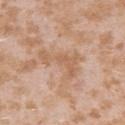The lesion was tiled from a total-body skin photograph and was not biopsied. Located on the arm. The tile uses white-light illumination. The lesion's longest dimension is about 5 mm. A lesion tile, about 15 mm wide, cut from a 3D total-body photograph. A female subject in their mid-20s. The lesion-visualizer software estimated a lesion area of about 9.5 mm², an eccentricity of roughly 0.85, and a shape-asymmetry score of about 0.65 (0 = symmetric). It also reported a within-lesion color-variation index near 1.5/10 and radial color variation of about 0.5. The analysis additionally found an automated nevus-likeness rating near 0 out of 100 and lesion-presence confidence of about 90/100.